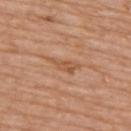Imaged during a routine full-body skin examination; the lesion was not biopsied and no histopathology is available.
On the upper back.
Automated image analysis of the tile measured about 7 CIELAB-L* units darker than the surrounding skin and a normalized border contrast of about 5.5.
Measured at roughly 4 mm in maximum diameter.
A region of skin cropped from a whole-body photographic capture, roughly 15 mm wide.
The patient is a female aged approximately 75.